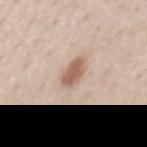Clinical impression: Recorded during total-body skin imaging; not selected for excision or biopsy. Clinical summary: The total-body-photography lesion software estimated about 13 CIELAB-L* units darker than the surrounding skin and a lesion-to-skin contrast of about 8.5 (normalized; higher = more distinct). A male subject, approximately 60 years of age. A roughly 15 mm field-of-view crop from a total-body skin photograph. On the abdomen. Imaged with white-light lighting. The recorded lesion diameter is about 3.5 mm.